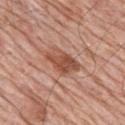Q: Was a biopsy performed?
A: catalogued during a skin exam; not biopsied
Q: Automated lesion metrics?
A: an area of roughly 9 mm² and a symmetry-axis asymmetry near 0.3; about 12 CIELAB-L* units darker than the surrounding skin and a normalized lesion–skin contrast near 8.5; a color-variation rating of about 4.5/10; a nevus-likeness score of about 60/100 and a detector confidence of about 100 out of 100 that the crop contains a lesion
Q: Patient demographics?
A: male, roughly 80 years of age
Q: What is the imaging modality?
A: 15 mm crop, total-body photography
Q: Lesion size?
A: ~4.5 mm (longest diameter)
Q: What lighting was used for the tile?
A: white-light
Q: Where on the body is the lesion?
A: the mid back